A male subject aged around 40 · the lesion is located on the right forearm · a lesion tile, about 15 mm wide, cut from a 3D total-body photograph.
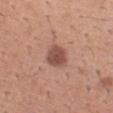The tile uses white-light illumination.
An algorithmic analysis of the crop reported a lesion color around L≈50 a*≈23 b*≈25 in CIELAB, roughly 12 lightness units darker than nearby skin, and a normalized lesion–skin contrast near 9.
Measured at roughly 3 mm in maximum diameter.
Biopsy histopathology demonstrated an atypical melanocytic neoplasm — an indeterminate (borderline) lesion.